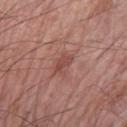• biopsy status — imaged on a skin check; not biopsied
• site — the left forearm
• image source — total-body-photography crop, ~15 mm field of view
• subject — male, aged around 65
• size — ≈2.5 mm